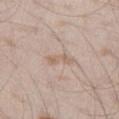biopsy_status: not biopsied; imaged during a skin examination
patient:
  sex: male
  age_approx: 45
image:
  source: total-body photography crop
  field_of_view_mm: 15
site: left thigh
lesion_size:
  long_diameter_mm_approx: 3.0
lighting: white-light